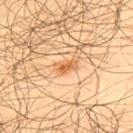lighting — cross-polarized | size — about 3 mm | patient — male, aged 63–67 | anatomic site — the upper back | image source — ~15 mm tile from a whole-body skin photo.